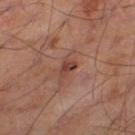| feature | finding |
|---|---|
| notes | no biopsy performed (imaged during a skin exam) |
| lesion diameter | ≈2 mm |
| imaging modality | 15 mm crop, total-body photography |
| anatomic site | the leg |
| subject | male, aged approximately 55 |